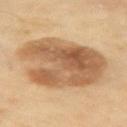Recorded during total-body skin imaging; not selected for excision or biopsy. The lesion is located on the left upper arm. Captured under cross-polarized illumination. A male patient about 70 years old. This image is a 15 mm lesion crop taken from a total-body photograph. Approximately 9 mm at its widest.